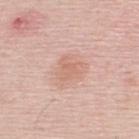  biopsy_status: not biopsied; imaged during a skin examination
  image:
    source: total-body photography crop
    field_of_view_mm: 15
  patient:
    sex: male
    age_approx: 50
  lesion_size:
    long_diameter_mm_approx: 3.0
  site: upper back
  automated_metrics:
    area_mm2_approx: 6.0
    shape_asymmetry: 0.45
    cielab_L: 65
    cielab_a: 22
    cielab_b: 28
    vs_skin_darker_L: 7.0
    nevus_likeness_0_100: 0
    lesion_detection_confidence_0_100: 100
  lighting: white-light